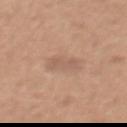Recorded during total-body skin imaging; not selected for excision or biopsy.
The lesion is on the abdomen.
A close-up tile cropped from a whole-body skin photograph, about 15 mm across.
The subject is a male in their mid- to late 50s.